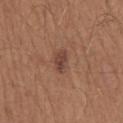{
  "biopsy_status": "not biopsied; imaged during a skin examination",
  "site": "mid back",
  "patient": {
    "sex": "male",
    "age_approx": 65
  },
  "image": {
    "source": "total-body photography crop",
    "field_of_view_mm": 15
  }
}